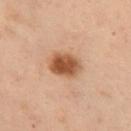Clinical impression: Recorded during total-body skin imaging; not selected for excision or biopsy. Clinical summary: Longest diameter approximately 4 mm. The patient is a female about 55 years old. The lesion is located on the front of the torso. A 15 mm crop from a total-body photograph taken for skin-cancer surveillance.